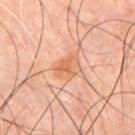This lesion was catalogued during total-body skin photography and was not selected for biopsy.
Captured under cross-polarized illumination.
A male patient aged approximately 45.
Approximately 3 mm at its widest.
The total-body-photography lesion software estimated a lesion color around L≈61 a*≈24 b*≈36 in CIELAB, about 8 CIELAB-L* units darker than the surrounding skin, and a normalized border contrast of about 6.5.
A roughly 15 mm field-of-view crop from a total-body skin photograph.
The lesion is on the chest.